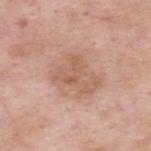<tbp_lesion>
  <biopsy_status>not biopsied; imaged during a skin examination</biopsy_status>
</tbp_lesion>